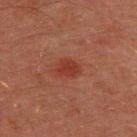Impression:
No biopsy was performed on this lesion — it was imaged during a full skin examination and was not determined to be concerning.
Clinical summary:
About 3 mm across. This is a cross-polarized tile. The patient is a male roughly 45 years of age. The lesion is located on the upper back. A region of skin cropped from a whole-body photographic capture, roughly 15 mm wide. Automated image analysis of the tile measured a border-irregularity index near 2/10 and a within-lesion color-variation index near 2/10.